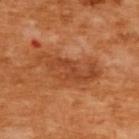Background:
The lesion is located on the upper back. Captured under cross-polarized illumination. About 7.5 mm across. The patient is a female aged around 55. Automated tile analysis of the lesion measured a border-irregularity rating of about 6.5/10, a color-variation rating of about 3.5/10, and peripheral color asymmetry of about 1. The software also gave a classifier nevus-likeness of about 0/100 and a detector confidence of about 100 out of 100 that the crop contains a lesion. A 15 mm close-up extracted from a 3D total-body photography capture.